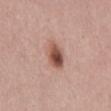Acquisition and patient details: Imaged with white-light lighting. The lesion-visualizer software estimated a footprint of about 6.5 mm², a shape eccentricity near 0.75, and a symmetry-axis asymmetry near 0.2. And it measured an average lesion color of about L≈51 a*≈22 b*≈27 (CIELAB) and roughly 15 lightness units darker than nearby skin. It also reported border irregularity of about 2 on a 0–10 scale and a within-lesion color-variation index near 8/10. A female subject, aged 63 to 67. On the abdomen. A 15 mm close-up extracted from a 3D total-body photography capture.